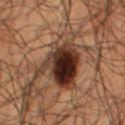Recorded during total-body skin imaging; not selected for excision or biopsy.